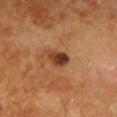Q: Was a biopsy performed?
A: catalogued during a skin exam; not biopsied
Q: Automated lesion metrics?
A: a footprint of about 5 mm², an outline eccentricity of about 0.65 (0 = round, 1 = elongated), and a symmetry-axis asymmetry near 0.25; roughly 12 lightness units darker than nearby skin and a normalized border contrast of about 10.5
Q: Where on the body is the lesion?
A: the chest
Q: How was this image acquired?
A: 15 mm crop, total-body photography
Q: Patient demographics?
A: female, about 50 years old